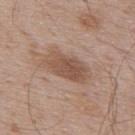Imaged during a routine full-body skin examination; the lesion was not biopsied and no histopathology is available. On the upper back. Automated tile analysis of the lesion measured an average lesion color of about L≈51 a*≈18 b*≈27 (CIELAB), roughly 10 lightness units darker than nearby skin, and a normalized lesion–skin contrast near 7.5. The analysis additionally found a nevus-likeness score of about 15/100 and a detector confidence of about 100 out of 100 that the crop contains a lesion. Imaged with white-light lighting. A lesion tile, about 15 mm wide, cut from a 3D total-body photograph. The patient is a male aged 68–72. The recorded lesion diameter is about 5 mm.